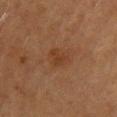Clinical impression: Part of a total-body skin-imaging series; this lesion was reviewed on a skin check and was not flagged for biopsy. Clinical summary: Imaged with cross-polarized lighting. Cropped from a total-body skin-imaging series; the visible field is about 15 mm. The lesion is located on the back. Approximately 2.5 mm at its widest. The lesion-visualizer software estimated a shape eccentricity near 0.6 and a shape-asymmetry score of about 0.35 (0 = symmetric). And it measured an average lesion color of about L≈34 a*≈20 b*≈30 (CIELAB) and about 5 CIELAB-L* units darker than the surrounding skin. It also reported a border-irregularity rating of about 3/10 and internal color variation of about 1.5 on a 0–10 scale. And it measured lesion-presence confidence of about 100/100. The patient is a female aged approximately 60.